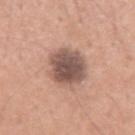No biopsy was performed on this lesion — it was imaged during a full skin examination and was not determined to be concerning.
An algorithmic analysis of the crop reported border irregularity of about 2 on a 0–10 scale, a within-lesion color-variation index near 4.5/10, and peripheral color asymmetry of about 1.
A close-up tile cropped from a whole-body skin photograph, about 15 mm across.
Longest diameter approximately 5 mm.
The patient is a male in their 30s.
From the arm.
This is a white-light tile.